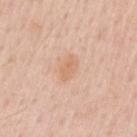Q: Was a biopsy performed?
A: no biopsy performed (imaged during a skin exam)
Q: How was the tile lit?
A: white-light
Q: Where on the body is the lesion?
A: the arm
Q: What are the patient's age and sex?
A: male, in their 60s
Q: What is the imaging modality?
A: 15 mm crop, total-body photography
Q: Lesion size?
A: ≈3 mm